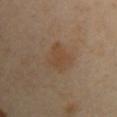follow-up — imaged on a skin check; not biopsied
lesion size — about 3.5 mm
patient — male, aged approximately 50
anatomic site — the right upper arm
lighting — cross-polarized illumination
automated metrics — a border-irregularity rating of about 3/10 and a within-lesion color-variation index near 1.5/10; a classifier nevus-likeness of about 55/100 and a lesion-detection confidence of about 100/100
image source — 15 mm crop, total-body photography